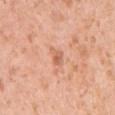Assessment:
The lesion was photographed on a routine skin check and not biopsied; there is no pathology result.
Clinical summary:
The patient is a female aged approximately 40. On the left upper arm. A 15 mm close-up tile from a total-body photography series done for melanoma screening.